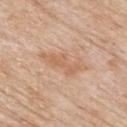The lesion was photographed on a routine skin check and not biopsied; there is no pathology result.
The patient is a male approximately 85 years of age or older.
The lesion is on the back.
A roughly 15 mm field-of-view crop from a total-body skin photograph.
This is a white-light tile.
Longest diameter approximately 5.5 mm.